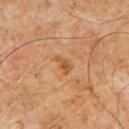notes: total-body-photography surveillance lesion; no biopsy | TBP lesion metrics: a lesion area of about 3 mm², a shape eccentricity near 0.8, and two-axis asymmetry of about 0.35; a within-lesion color-variation index near 1.5/10 and peripheral color asymmetry of about 0.5 | subject: male, aged 58–62 | location: the chest | image source: ~15 mm tile from a whole-body skin photo.